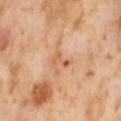{
  "biopsy_status": "not biopsied; imaged during a skin examination",
  "patient": {
    "sex": "female",
    "age_approx": 55
  },
  "image": {
    "source": "total-body photography crop",
    "field_of_view_mm": 15
  },
  "site": "abdomen"
}